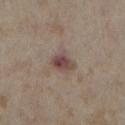Q: How large is the lesion?
A: ~2.5 mm (longest diameter)
Q: Illumination type?
A: cross-polarized
Q: Automated lesion metrics?
A: an area of roughly 4.5 mm², an outline eccentricity of about 0.65 (0 = round, 1 = elongated), and a shape-asymmetry score of about 0.3 (0 = symmetric); a border-irregularity rating of about 2.5/10 and internal color variation of about 5.5 on a 0–10 scale; a lesion-detection confidence of about 100/100
Q: Where on the body is the lesion?
A: the left lower leg
Q: What are the patient's age and sex?
A: female, aged approximately 35
Q: How was this image acquired?
A: ~15 mm tile from a whole-body skin photo